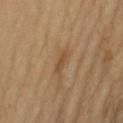lighting: cross-polarized | patient: female, about 55 years old | lesion diameter: ~2.5 mm (longest diameter) | automated metrics: a lesion area of about 3 mm²; a classifier nevus-likeness of about 0/100 and a detector confidence of about 100 out of 100 that the crop contains a lesion | imaging modality: ~15 mm tile from a whole-body skin photo | site: the right upper arm.